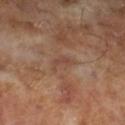The lesion was photographed on a routine skin check and not biopsied; there is no pathology result. Cropped from a total-body skin-imaging series; the visible field is about 15 mm. Automated tile analysis of the lesion measured an area of roughly 3.5 mm² and an eccentricity of roughly 0.85. The analysis additionally found a lesion color around L≈43 a*≈18 b*≈26 in CIELAB, about 6 CIELAB-L* units darker than the surrounding skin, and a normalized border contrast of about 5. It also reported a border-irregularity index near 5.5/10 and a peripheral color-asymmetry measure near 0.5. A male subject, roughly 65 years of age.